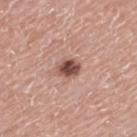{
  "biopsy_status": "not biopsied; imaged during a skin examination",
  "site": "mid back",
  "image": {
    "source": "total-body photography crop",
    "field_of_view_mm": 15
  },
  "patient": {
    "sex": "male",
    "age_approx": 75
  }
}